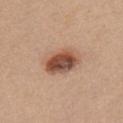The lesion is on the chest. This is a white-light tile. The total-body-photography lesion software estimated a lesion color around L≈51 a*≈22 b*≈30 in CIELAB, roughly 15 lightness units darker than nearby skin, and a lesion-to-skin contrast of about 10.5 (normalized; higher = more distinct). And it measured a color-variation rating of about 8/10 and a peripheral color-asymmetry measure near 2.5. The patient is a female aged 43–47. About 4.5 mm across. A region of skin cropped from a whole-body photographic capture, roughly 15 mm wide.